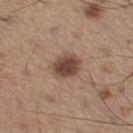image: ~15 mm tile from a whole-body skin photo; anatomic site: the left thigh; patient: male, approximately 65 years of age.